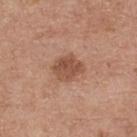Q: Was this lesion biopsied?
A: no biopsy performed (imaged during a skin exam)